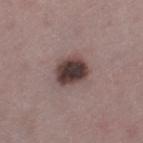{
  "biopsy_status": "not biopsied; imaged during a skin examination",
  "automated_metrics": {
    "color_variation_0_10": 6.5,
    "peripheral_color_asymmetry": 1.5,
    "nevus_likeness_0_100": 50,
    "lesion_detection_confidence_0_100": 100
  },
  "image": {
    "source": "total-body photography crop",
    "field_of_view_mm": 15
  },
  "lesion_size": {
    "long_diameter_mm_approx": 4.0
  },
  "lighting": "white-light",
  "patient": {
    "sex": "female",
    "age_approx": 45
  },
  "site": "left thigh"
}